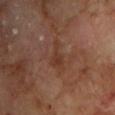Q: Is there a histopathology result?
A: catalogued during a skin exam; not biopsied
Q: How was this image acquired?
A: ~15 mm crop, total-body skin-cancer survey
Q: Patient demographics?
A: male, roughly 70 years of age
Q: Lesion location?
A: the chest